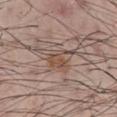The lesion was tiled from a total-body skin photograph and was not biopsied.
Captured under white-light illumination.
The total-body-photography lesion software estimated a footprint of about 8 mm², an eccentricity of roughly 0.75, and a symmetry-axis asymmetry near 0.4.
A lesion tile, about 15 mm wide, cut from a 3D total-body photograph.
Measured at roughly 4.5 mm in maximum diameter.
A male patient, in their 60s.
On the chest.